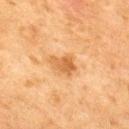biopsy_status: not biopsied; imaged during a skin examination
lighting: cross-polarized
lesion_size:
  long_diameter_mm_approx: 3.5
image:
  source: total-body photography crop
  field_of_view_mm: 15
automated_metrics:
  cielab_L: 50
  cielab_a: 21
  cielab_b: 38
  vs_skin_contrast_norm: 7.0
  border_irregularity_0_10: 4.0
  color_variation_0_10: 3.0
  nevus_likeness_0_100: 65
  lesion_detection_confidence_0_100: 100
site: upper back
patient:
  sex: male
  age_approx: 50